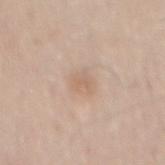This is a white-light tile. A 15 mm close-up extracted from a 3D total-body photography capture. About 3 mm across. Located on the mid back. Automated image analysis of the tile measured a lesion color around L≈64 a*≈16 b*≈29 in CIELAB, about 6 CIELAB-L* units darker than the surrounding skin, and a normalized lesion–skin contrast near 4.5. And it measured a border-irregularity index near 2.5/10, a within-lesion color-variation index near 2/10, and peripheral color asymmetry of about 0.5. And it measured an automated nevus-likeness rating near 0 out of 100. A male subject roughly 50 years of age.